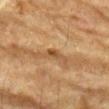A 15 mm close-up extracted from a 3D total-body photography capture. From the left forearm. Longest diameter approximately 2.5 mm. A male patient, approximately 85 years of age or older.